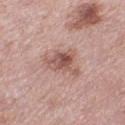No biopsy was performed on this lesion — it was imaged during a full skin examination and was not determined to be concerning. Cropped from a whole-body photographic skin survey; the tile spans about 15 mm. From the leg. About 4.5 mm across. The subject is a female aged around 40. The tile uses white-light illumination.